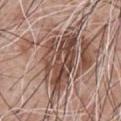– notes — imaged on a skin check; not biopsied
– lighting — white-light
– image — total-body-photography crop, ~15 mm field of view
– location — the chest
– patient — male, aged around 60
– TBP lesion metrics — an area of roughly 22 mm², a shape eccentricity near 0.8, and a shape-asymmetry score of about 0.35 (0 = symmetric); an average lesion color of about L≈47 a*≈19 b*≈25 (CIELAB) and a lesion-to-skin contrast of about 9.5 (normalized; higher = more distinct); a border-irregularity index near 6.5/10 and internal color variation of about 7.5 on a 0–10 scale; a nevus-likeness score of about 15/100 and a lesion-detection confidence of about 65/100